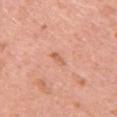The lesion was tiled from a total-body skin photograph and was not biopsied. Automated image analysis of the tile measured a lesion area of about 2 mm², an outline eccentricity of about 0.9 (0 = round, 1 = elongated), and a symmetry-axis asymmetry near 0.35. It also reported a detector confidence of about 100 out of 100 that the crop contains a lesion. Measured at roughly 2.5 mm in maximum diameter. A female subject, roughly 45 years of age. A lesion tile, about 15 mm wide, cut from a 3D total-body photograph. On the upper back.